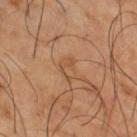Acquisition and patient details:
A male patient, aged approximately 65. Located on the right thigh. A lesion tile, about 15 mm wide, cut from a 3D total-body photograph. This is a cross-polarized tile. The recorded lesion diameter is about 2.5 mm.